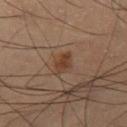Assessment: Part of a total-body skin-imaging series; this lesion was reviewed on a skin check and was not flagged for biopsy. Context: The lesion is on the right thigh. A 15 mm crop from a total-body photograph taken for skin-cancer surveillance. The patient is a male aged around 65.